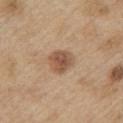| key | value |
|---|---|
| size | about 3.5 mm |
| image | 15 mm crop, total-body photography |
| site | the arm |
| TBP lesion metrics | an automated nevus-likeness rating near 75 out of 100 and lesion-presence confidence of about 100/100 |
| patient | male, aged approximately 70 |
| lighting | white-light |